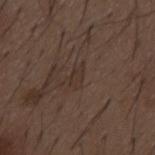Recorded during total-body skin imaging; not selected for excision or biopsy. From the mid back. A male patient aged 48 to 52. A region of skin cropped from a whole-body photographic capture, roughly 15 mm wide.